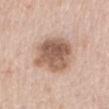patient:
  sex: female
  age_approx: 50
image:
  source: total-body photography crop
  field_of_view_mm: 15
site: mid back